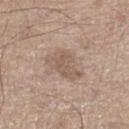Assessment:
Part of a total-body skin-imaging series; this lesion was reviewed on a skin check and was not flagged for biopsy.
Context:
A lesion tile, about 15 mm wide, cut from a 3D total-body photograph. Captured under white-light illumination. The recorded lesion diameter is about 4.5 mm. A male subject aged 68 to 72. From the left lower leg.